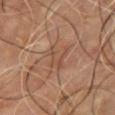Findings:
• biopsy status — imaged on a skin check; not biopsied
• image-analysis metrics — an area of roughly 6 mm² and a shape eccentricity near 0.65; a mean CIELAB color near L≈46 a*≈19 b*≈28, roughly 6 lightness units darker than nearby skin, and a normalized border contrast of about 5; a border-irregularity index near 8/10, a color-variation rating of about 3/10, and radial color variation of about 1; a classifier nevus-likeness of about 0/100 and lesion-presence confidence of about 75/100
• acquisition — 15 mm crop, total-body photography
• subject — male, in their mid- to late 60s
• lighting — cross-polarized
• location — the chest
• lesion diameter — ~3.5 mm (longest diameter)